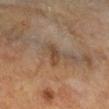{
  "biopsy_status": "not biopsied; imaged during a skin examination",
  "lesion_size": {
    "long_diameter_mm_approx": 3.5
  },
  "image": {
    "source": "total-body photography crop",
    "field_of_view_mm": 15
  },
  "automated_metrics": {
    "vs_skin_contrast_norm": 7.0,
    "border_irregularity_0_10": 4.5,
    "color_variation_0_10": 1.5,
    "peripheral_color_asymmetry": 0.5,
    "nevus_likeness_0_100": 0
  },
  "site": "right lower leg",
  "lighting": "cross-polarized",
  "patient": {
    "sex": "male",
    "age_approx": 60
  }
}